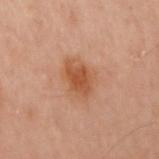workup — no biopsy performed (imaged during a skin exam)
acquisition — ~15 mm crop, total-body skin-cancer survey
image-analysis metrics — an area of roughly 12 mm² and an eccentricity of roughly 0.65; about 8 CIELAB-L* units darker than the surrounding skin
site — the left arm
patient — female, aged approximately 60
illumination — cross-polarized
diameter — ≈4.5 mm